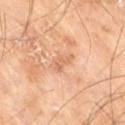Assessment: This lesion was catalogued during total-body skin photography and was not selected for biopsy. Context: A 15 mm close-up extracted from a 3D total-body photography capture. A male patient aged 68 to 72. From the right thigh. The lesion's longest dimension is about 3 mm.